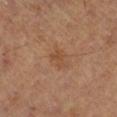  biopsy_status: not biopsied; imaged during a skin examination
  image:
    source: total-body photography crop
    field_of_view_mm: 15
  patient:
    sex: male
    age_approx: 60
  lesion_size:
    long_diameter_mm_approx: 2.5
  lighting: cross-polarized
  automated_metrics:
    shape_asymmetry: 0.35
    cielab_L: 45
    cielab_a: 20
    cielab_b: 31
    vs_skin_darker_L: 6.0
    vs_skin_contrast_norm: 5.5
    border_irregularity_0_10: 3.5
    color_variation_0_10: 0.5
    peripheral_color_asymmetry: 0.0
  site: right lower leg